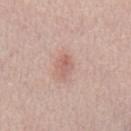notes: no biopsy performed (imaged during a skin exam)
anatomic site: the chest
imaging modality: ~15 mm crop, total-body skin-cancer survey
subject: male, aged around 40